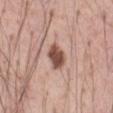{
  "biopsy_status": "not biopsied; imaged during a skin examination",
  "lighting": "white-light",
  "site": "abdomen",
  "image": {
    "source": "total-body photography crop",
    "field_of_view_mm": 15
  },
  "automated_metrics": {
    "cielab_L": 50,
    "cielab_a": 21,
    "cielab_b": 26,
    "vs_skin_darker_L": 16.0,
    "vs_skin_contrast_norm": 11.0,
    "border_irregularity_0_10": 2.5,
    "color_variation_0_10": 3.5,
    "peripheral_color_asymmetry": 1.0
  },
  "patient": {
    "sex": "male",
    "age_approx": 55
  },
  "lesion_size": {
    "long_diameter_mm_approx": 3.5
  }
}